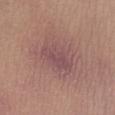Part of a total-body skin-imaging series; this lesion was reviewed on a skin check and was not flagged for biopsy. A female patient aged around 25. Cropped from a whole-body photographic skin survey; the tile spans about 15 mm. On the left lower leg.